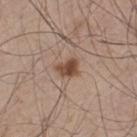follow-up: catalogued during a skin exam; not biopsied | patient: male, in their mid-40s | automated lesion analysis: two-axis asymmetry of about 0.35; border irregularity of about 3.5 on a 0–10 scale, a within-lesion color-variation index near 4/10, and peripheral color asymmetry of about 1.5; a classifier nevus-likeness of about 95/100 and lesion-presence confidence of about 100/100 | tile lighting: white-light illumination | lesion diameter: ≈3 mm | image source: total-body-photography crop, ~15 mm field of view | location: the leg.